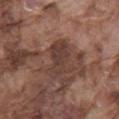Q: Is there a histopathology result?
A: catalogued during a skin exam; not biopsied
Q: Lesion location?
A: the mid back
Q: What is the lesion's diameter?
A: ~5 mm (longest diameter)
Q: How was this image acquired?
A: ~15 mm crop, total-body skin-cancer survey
Q: Patient demographics?
A: male, approximately 75 years of age